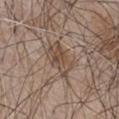This lesion was catalogued during total-body skin photography and was not selected for biopsy. This is a white-light tile. Located on the chest. A male patient approximately 65 years of age. Cropped from a total-body skin-imaging series; the visible field is about 15 mm.